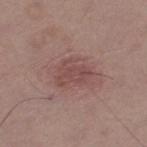The lesion was tiled from a total-body skin photograph and was not biopsied. The lesion is on the left thigh. A male patient, aged 68–72. A close-up tile cropped from a whole-body skin photograph, about 15 mm across.